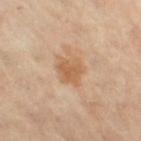biopsy status: imaged on a skin check; not biopsied | subject: female, in their mid-70s | TBP lesion metrics: a lesion area of about 6.5 mm² and a symmetry-axis asymmetry near 0.2; a color-variation rating of about 2.5/10 and a peripheral color-asymmetry measure near 1; an automated nevus-likeness rating near 15 out of 100 and a detector confidence of about 100 out of 100 that the crop contains a lesion | acquisition: ~15 mm tile from a whole-body skin photo | body site: the left thigh | size: about 3.5 mm.